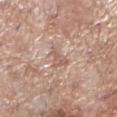biopsy status = imaged on a skin check; not biopsied | illumination = white-light | automated lesion analysis = an average lesion color of about L≈59 a*≈18 b*≈26 (CIELAB), about 9 CIELAB-L* units darker than the surrounding skin, and a normalized border contrast of about 5.5 | imaging modality = ~15 mm crop, total-body skin-cancer survey | patient = female, aged 73 to 77 | size = about 2.5 mm | location = the left lower leg.